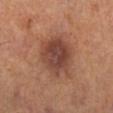Part of a total-body skin-imaging series; this lesion was reviewed on a skin check and was not flagged for biopsy.
The lesion-visualizer software estimated a symmetry-axis asymmetry near 0.2. And it measured an average lesion color of about L≈43 a*≈22 b*≈27 (CIELAB) and a normalized border contrast of about 8.5. The software also gave border irregularity of about 2 on a 0–10 scale and internal color variation of about 4.5 on a 0–10 scale.
The tile uses cross-polarized illumination.
A region of skin cropped from a whole-body photographic capture, roughly 15 mm wide.
A female subject roughly 40 years of age.
Located on the right lower leg.